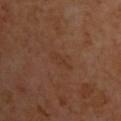Acquisition and patient details: A close-up tile cropped from a whole-body skin photograph, about 15 mm across. Located on the upper back. The tile uses cross-polarized illumination. A male subject, in their mid- to late 60s. Automated image analysis of the tile measured a border-irregularity rating of about 5/10 and a within-lesion color-variation index near 0/10.